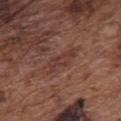Case summary:
– biopsy status: no biopsy performed (imaged during a skin exam)
– subject: male, in their mid- to late 70s
– automated metrics: an outline eccentricity of about 0.95 (0 = round, 1 = elongated); a normalized border contrast of about 5
– body site: the front of the torso
– illumination: white-light illumination
– acquisition: ~15 mm tile from a whole-body skin photo